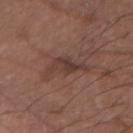No biopsy was performed on this lesion — it was imaged during a full skin examination and was not determined to be concerning. A male subject, roughly 65 years of age. On the right forearm. A 15 mm close-up tile from a total-body photography series done for melanoma screening. The tile uses white-light illumination.